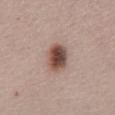- notes — imaged on a skin check; not biopsied
- TBP lesion metrics — a border-irregularity rating of about 1/10, a within-lesion color-variation index near 8.5/10, and radial color variation of about 2.5
- image source — ~15 mm tile from a whole-body skin photo
- subject — female, aged approximately 65
- body site — the abdomen
- tile lighting — white-light illumination